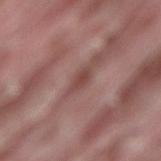Findings:
• notes · imaged on a skin check; not biopsied
• imaging modality · 15 mm crop, total-body photography
• tile lighting · white-light illumination
• diameter · ≈3 mm
• subject · male, aged around 40
• anatomic site · the back